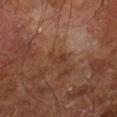Impression:
Imaged during a routine full-body skin examination; the lesion was not biopsied and no histopathology is available.
Acquisition and patient details:
A 15 mm crop from a total-body photograph taken for skin-cancer surveillance. Longest diameter approximately 2.5 mm. The lesion is on the right leg. A male patient, aged approximately 60. Automated tile analysis of the lesion measured a border-irregularity index near 5.5/10, internal color variation of about 0 on a 0–10 scale, and peripheral color asymmetry of about 0.